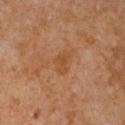notes: imaged on a skin check; not biopsied
subject: female, aged approximately 50
image: ~15 mm tile from a whole-body skin photo
image-analysis metrics: a footprint of about 4.5 mm² and two-axis asymmetry of about 0.4; an automated nevus-likeness rating near 0 out of 100 and a detector confidence of about 100 out of 100 that the crop contains a lesion
location: the chest